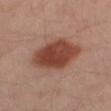notes: catalogued during a skin exam; not biopsied | location: the leg | size: ≈7 mm | image source: total-body-photography crop, ~15 mm field of view | patient: male, in their mid-50s | lighting: cross-polarized illumination.